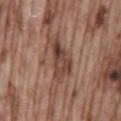Part of a total-body skin-imaging series; this lesion was reviewed on a skin check and was not flagged for biopsy.
Measured at roughly 5 mm in maximum diameter.
On the mid back.
A region of skin cropped from a whole-body photographic capture, roughly 15 mm wide.
A male subject aged approximately 75.
This is a white-light tile.